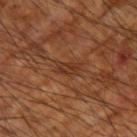The patient is a male aged around 60. A close-up tile cropped from a whole-body skin photograph, about 15 mm across. The lesion is located on the right upper arm.